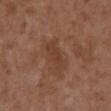Q: Was a biopsy performed?
A: catalogued during a skin exam; not biopsied
Q: How was this image acquired?
A: ~15 mm tile from a whole-body skin photo
Q: What lighting was used for the tile?
A: white-light
Q: What are the patient's age and sex?
A: male, aged 73–77
Q: What is the anatomic site?
A: the abdomen
Q: What did automated image analysis measure?
A: an average lesion color of about L≈39 a*≈21 b*≈27 (CIELAB) and roughly 6 lightness units darker than nearby skin; a within-lesion color-variation index near 2.5/10 and radial color variation of about 1
Q: Lesion size?
A: ~3.5 mm (longest diameter)